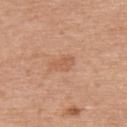  biopsy_status: not biopsied; imaged during a skin examination
  site: upper back
  image:
    source: total-body photography crop
    field_of_view_mm: 15
  patient:
    sex: male
    age_approx: 80